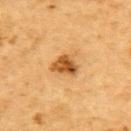Findings:
* workup — total-body-photography surveillance lesion; no biopsy
* anatomic site — the upper back
* lighting — cross-polarized illumination
* patient — male, aged around 85 or older
* image — ~15 mm crop, total-body skin-cancer survey